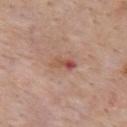image source: ~15 mm crop, total-body skin-cancer survey | tile lighting: white-light illumination | lesion diameter: about 3 mm | TBP lesion metrics: a lesion area of about 4 mm², a shape eccentricity near 0.9, and a symmetry-axis asymmetry near 0.4; internal color variation of about 10 on a 0–10 scale and radial color variation of about 4; a classifier nevus-likeness of about 0/100 and a lesion-detection confidence of about 100/100 | location: the mid back | subject: male, aged 63 to 67.